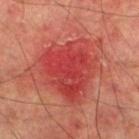workup: catalogued during a skin exam; not biopsied | image: ~15 mm tile from a whole-body skin photo | site: the left lower leg | lesion size: ~7 mm (longest diameter) | subject: male, aged approximately 75 | TBP lesion metrics: a lesion area of about 29 mm² and a shape-asymmetry score of about 0.35 (0 = symmetric); an average lesion color of about L≈38 a*≈36 b*≈27 (CIELAB), about 8 CIELAB-L* units darker than the surrounding skin, and a normalized lesion–skin contrast near 7; a border-irregularity rating of about 4/10, internal color variation of about 5 on a 0–10 scale, and peripheral color asymmetry of about 1.5; a detector confidence of about 100 out of 100 that the crop contains a lesion | illumination: cross-polarized.